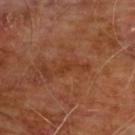workup: catalogued during a skin exam; not biopsied
imaging modality: ~15 mm crop, total-body skin-cancer survey
location: the chest
illumination: cross-polarized illumination
lesion size: about 2.5 mm
automated lesion analysis: border irregularity of about 3.5 on a 0–10 scale and a peripheral color-asymmetry measure near 0; an automated nevus-likeness rating near 0 out of 100 and a lesion-detection confidence of about 95/100
patient: male, aged approximately 60